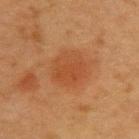Notes:
* patient — female, in their 40s
* body site — the upper back
* image — ~15 mm tile from a whole-body skin photo